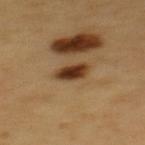Impression:
Part of a total-body skin-imaging series; this lesion was reviewed on a skin check and was not flagged for biopsy.
Background:
The lesion is located on the back. A male subject, aged around 60. A 15 mm close-up tile from a total-body photography series done for melanoma screening. The recorded lesion diameter is about 3 mm. Captured under cross-polarized illumination.